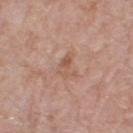anatomic site = the right thigh | acquisition = ~15 mm tile from a whole-body skin photo | subject = female, about 70 years old.